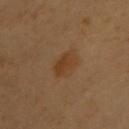Q: Lesion location?
A: the upper back
Q: What kind of image is this?
A: ~15 mm crop, total-body skin-cancer survey
Q: Patient demographics?
A: female, aged approximately 60